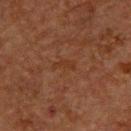follow-up: total-body-photography surveillance lesion; no biopsy
patient: male, aged 58–62
lighting: cross-polarized
image: ~15 mm crop, total-body skin-cancer survey
site: the upper back
image-analysis metrics: an area of roughly 3 mm², an eccentricity of roughly 0.9, and a symmetry-axis asymmetry near 0.45; a mean CIELAB color near L≈26 a*≈18 b*≈25, a lesion–skin lightness drop of about 3, and a lesion-to-skin contrast of about 4.5 (normalized; higher = more distinct); a nevus-likeness score of about 0/100
lesion size: ≈3 mm